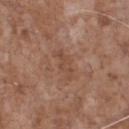Clinical impression:
The lesion was photographed on a routine skin check and not biopsied; there is no pathology result.
Clinical summary:
A male subject, aged 68–72. A 15 mm close-up extracted from a 3D total-body photography capture. From the front of the torso.